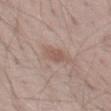* workup — total-body-photography surveillance lesion; no biopsy
* lesion size — ≈3 mm
* image source — total-body-photography crop, ~15 mm field of view
* body site — the leg
* subject — male, about 35 years old
* tile lighting — white-light illumination